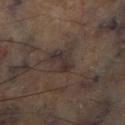The lesion was photographed on a routine skin check and not biopsied; there is no pathology result. The lesion-visualizer software estimated a lesion area of about 2.5 mm² and a shape-asymmetry score of about 0.55 (0 = symmetric). And it measured a classifier nevus-likeness of about 0/100 and lesion-presence confidence of about 85/100. Approximately 3 mm at its widest. On the right lower leg. The tile uses cross-polarized illumination. A region of skin cropped from a whole-body photographic capture, roughly 15 mm wide.